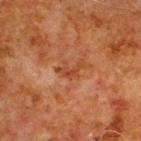{
  "biopsy_status": "not biopsied; imaged during a skin examination",
  "automated_metrics": {
    "area_mm2_approx": 3.0,
    "eccentricity": 0.9,
    "shape_asymmetry": 0.8,
    "border_irregularity_0_10": 8.0
  },
  "image": {
    "source": "total-body photography crop",
    "field_of_view_mm": 15
  },
  "site": "upper back",
  "patient": {
    "sex": "male",
    "age_approx": 80
  },
  "lesion_size": {
    "long_diameter_mm_approx": 3.5
  },
  "lighting": "cross-polarized"
}